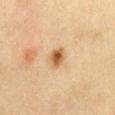The lesion was photographed on a routine skin check and not biopsied; there is no pathology result. The lesion-visualizer software estimated a lesion area of about 4.5 mm², a shape eccentricity near 0.6, and two-axis asymmetry of about 0.2. And it measured an average lesion color of about L≈54 a*≈18 b*≈38 (CIELAB) and roughly 13 lightness units darker than nearby skin. The analysis additionally found border irregularity of about 2 on a 0–10 scale and peripheral color asymmetry of about 1.5. A female subject, aged approximately 30. About 2.5 mm across. On the chest. A 15 mm close-up extracted from a 3D total-body photography capture.